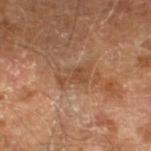The lesion was photographed on a routine skin check and not biopsied; there is no pathology result. Imaged with cross-polarized lighting. A close-up tile cropped from a whole-body skin photograph, about 15 mm across. Approximately 3.5 mm at its widest. A male patient, aged around 70. The lesion is on the leg.